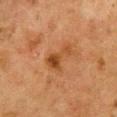Q: Was a biopsy performed?
A: catalogued during a skin exam; not biopsied
Q: How large is the lesion?
A: about 3.5 mm
Q: Illumination type?
A: cross-polarized illumination
Q: What did automated image analysis measure?
A: an area of roughly 6 mm², a shape eccentricity near 0.85, and a symmetry-axis asymmetry near 0.6; a lesion color around L≈39 a*≈22 b*≈34 in CIELAB, a lesion–skin lightness drop of about 8, and a normalized lesion–skin contrast near 7.5; border irregularity of about 6.5 on a 0–10 scale, internal color variation of about 3 on a 0–10 scale, and a peripheral color-asymmetry measure near 0.5; a classifier nevus-likeness of about 20/100 and lesion-presence confidence of about 100/100
Q: What is the imaging modality?
A: total-body-photography crop, ~15 mm field of view
Q: Patient demographics?
A: female, aged approximately 50
Q: Where on the body is the lesion?
A: the front of the torso